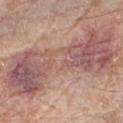No biopsy was performed on this lesion — it was imaged during a full skin examination and was not determined to be concerning.
Longest diameter approximately 14 mm.
The lesion-visualizer software estimated a border-irregularity index near 7.5/10, a color-variation rating of about 7.5/10, and a peripheral color-asymmetry measure near 2.5.
The lesion is on the right forearm.
Cropped from a whole-body photographic skin survey; the tile spans about 15 mm.
The patient is a male roughly 80 years of age.
This is a white-light tile.